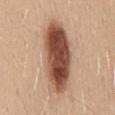workup: imaged on a skin check; not biopsied | lighting: white-light | subject: female, about 45 years old | lesion size: about 10 mm | automated lesion analysis: an area of roughly 29 mm², an outline eccentricity of about 0.9 (0 = round, 1 = elongated), and a shape-asymmetry score of about 0.15 (0 = symmetric); an average lesion color of about L≈50 a*≈22 b*≈29 (CIELAB) and a normalized lesion–skin contrast near 13; a classifier nevus-likeness of about 100/100 and lesion-presence confidence of about 100/100 | location: the mid back | imaging modality: ~15 mm crop, total-body skin-cancer survey.